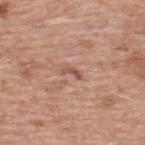follow-up: imaged on a skin check; not biopsied
location: the upper back
image source: ~15 mm crop, total-body skin-cancer survey
lesion diameter: ≈2.5 mm
tile lighting: white-light illumination
patient: male, in their mid-50s
TBP lesion metrics: an area of roughly 2 mm²; a border-irregularity rating of about 5/10, internal color variation of about 0 on a 0–10 scale, and peripheral color asymmetry of about 0; an automated nevus-likeness rating near 0 out of 100 and a detector confidence of about 95 out of 100 that the crop contains a lesion